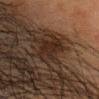This lesion was catalogued during total-body skin photography and was not selected for biopsy. The patient is a female aged 48 to 52. The total-body-photography lesion software estimated a lesion color around L≈21 a*≈13 b*≈20 in CIELAB, about 6 CIELAB-L* units darker than the surrounding skin, and a normalized border contrast of about 8. The analysis additionally found border irregularity of about 5 on a 0–10 scale, a color-variation rating of about 3/10, and radial color variation of about 1. The software also gave a nevus-likeness score of about 40/100 and lesion-presence confidence of about 55/100. The lesion's longest dimension is about 6 mm. Cropped from a total-body skin-imaging series; the visible field is about 15 mm. The tile uses cross-polarized illumination. On the head or neck.